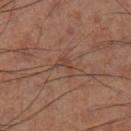{
  "biopsy_status": "not biopsied; imaged during a skin examination",
  "patient": {
    "sex": "male",
    "age_approx": 60
  },
  "lesion_size": {
    "long_diameter_mm_approx": 2.5
  },
  "site": "leg",
  "image": {
    "source": "total-body photography crop",
    "field_of_view_mm": 15
  }
}